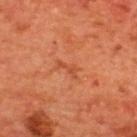{"biopsy_status": "not biopsied; imaged during a skin examination", "automated_metrics": {"eccentricity": 0.9, "shape_asymmetry": 0.6, "border_irregularity_0_10": 6.5, "color_variation_0_10": 0.0, "peripheral_color_asymmetry": 0.0}, "lighting": "cross-polarized", "lesion_size": {"long_diameter_mm_approx": 3.0}, "patient": {"sex": "male", "age_approx": 65}, "image": {"source": "total-body photography crop", "field_of_view_mm": 15}, "site": "upper back"}